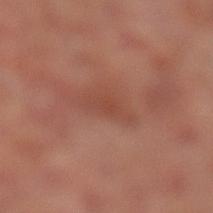workup=no biopsy performed (imaged during a skin exam); site=the left lower leg; imaging modality=~15 mm crop, total-body skin-cancer survey; lesion diameter=~3.5 mm (longest diameter); tile lighting=cross-polarized illumination; patient=male, aged 63 to 67.